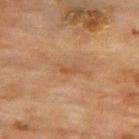biopsy_status: not biopsied; imaged during a skin examination